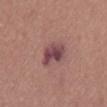Part of a total-body skin-imaging series; this lesion was reviewed on a skin check and was not flagged for biopsy.
The lesion's longest dimension is about 4.5 mm.
Cropped from a whole-body photographic skin survey; the tile spans about 15 mm.
A female subject, about 50 years old.
The total-body-photography lesion software estimated border irregularity of about 2.5 on a 0–10 scale and peripheral color asymmetry of about 2. The software also gave a nevus-likeness score of about 0/100 and a lesion-detection confidence of about 100/100.
The lesion is on the abdomen.
Imaged with white-light lighting.